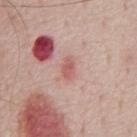The lesion was tiled from a total-body skin photograph and was not biopsied.
Located on the chest.
A male subject approximately 70 years of age.
A 15 mm close-up tile from a total-body photography series done for melanoma screening.